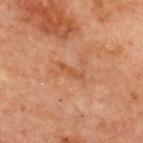biopsy status — catalogued during a skin exam; not biopsied | automated lesion analysis — a lesion color around L≈42 a*≈21 b*≈31 in CIELAB, a lesion–skin lightness drop of about 6, and a lesion-to-skin contrast of about 5.5 (normalized; higher = more distinct); internal color variation of about 0 on a 0–10 scale and a peripheral color-asymmetry measure near 0; an automated nevus-likeness rating near 0 out of 100 and a lesion-detection confidence of about 100/100 | location — the front of the torso | imaging modality — ~15 mm crop, total-body skin-cancer survey | lesion size — ~3 mm (longest diameter) | lighting — cross-polarized illumination | subject — male, about 65 years old.